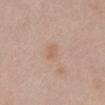Part of a total-body skin-imaging series; this lesion was reviewed on a skin check and was not flagged for biopsy. Captured under white-light illumination. The subject is a female aged 48 to 52. A roughly 15 mm field-of-view crop from a total-body skin photograph. Approximately 2.5 mm at its widest. The lesion is on the mid back.